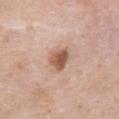Findings:
– workup: catalogued during a skin exam; not biopsied
– location: the chest
– tile lighting: white-light illumination
– lesion size: ~3.5 mm (longest diameter)
– subject: female, aged 58–62
– imaging modality: total-body-photography crop, ~15 mm field of view